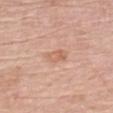<lesion>
  <biopsy_status>not biopsied; imaged during a skin examination</biopsy_status>
  <lesion_size>
    <long_diameter_mm_approx>3.0</long_diameter_mm_approx>
  </lesion_size>
  <automated_metrics>
    <cielab_L>63</cielab_L>
    <cielab_a>22</cielab_a>
    <cielab_b>32</cielab_b>
    <vs_skin_darker_L>7.0</vs_skin_darker_L>
    <vs_skin_contrast_norm>5.5</vs_skin_contrast_norm>
    <color_variation_0_10>2.5</color_variation_0_10>
    <peripheral_color_asymmetry>1.0</peripheral_color_asymmetry>
  </automated_metrics>
  <site>chest</site>
  <image>
    <source>total-body photography crop</source>
    <field_of_view_mm>15</field_of_view_mm>
  </image>
  <patient>
    <sex>female</sex>
    <age_approx>65</age_approx>
  </patient>
</lesion>